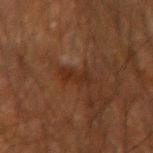Clinical impression: No biopsy was performed on this lesion — it was imaged during a full skin examination and was not determined to be concerning. Clinical summary: A close-up tile cropped from a whole-body skin photograph, about 15 mm across. Automated tile analysis of the lesion measured a footprint of about 3.5 mm², a shape eccentricity near 0.95, and a shape-asymmetry score of about 0.4 (0 = symmetric). The subject is a male approximately 60 years of age. Approximately 3.5 mm at its widest. The lesion is on the arm.